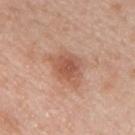<tbp_lesion>
<biopsy_status>not biopsied; imaged during a skin examination</biopsy_status>
<automated_metrics>
  <eccentricity>0.65</eccentricity>
  <shape_asymmetry>0.3</shape_asymmetry>
  <cielab_L>56</cielab_L>
  <cielab_a>23</cielab_a>
  <cielab_b>31</cielab_b>
  <vs_skin_darker_L>10.0</vs_skin_darker_L>
  <vs_skin_contrast_norm>7.0</vs_skin_contrast_norm>
  <border_irregularity_0_10>3.5</border_irregularity_0_10>
  <color_variation_0_10>3.0</color_variation_0_10>
  <peripheral_color_asymmetry>1.0</peripheral_color_asymmetry>
</automated_metrics>
<patient>
  <sex>male</sex>
  <age_approx>45</age_approx>
</patient>
<lighting>white-light</lighting>
<image>
  <source>total-body photography crop</source>
  <field_of_view_mm>15</field_of_view_mm>
</image>
<site>front of the torso</site>
</tbp_lesion>